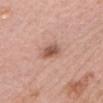follow-up=imaged on a skin check; not biopsied
tile lighting=white-light
site=the head or neck
image-analysis metrics=a footprint of about 5 mm²; an average lesion color of about L≈55 a*≈22 b*≈28 (CIELAB), a lesion–skin lightness drop of about 12, and a normalized lesion–skin contrast near 8; an automated nevus-likeness rating near 90 out of 100
image=~15 mm tile from a whole-body skin photo
patient=female, aged approximately 60
lesion diameter=about 2.5 mm